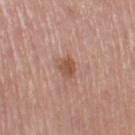Recorded during total-body skin imaging; not selected for excision or biopsy. This is a white-light tile. The lesion's longest dimension is about 2.5 mm. From the left thigh. A female patient, aged 63–67. Cropped from a whole-body photographic skin survey; the tile spans about 15 mm. Automated image analysis of the tile measured an area of roughly 4.5 mm², a shape eccentricity near 0.7, and a symmetry-axis asymmetry near 0.25. The software also gave a lesion–skin lightness drop of about 10 and a lesion-to-skin contrast of about 7 (normalized; higher = more distinct). It also reported border irregularity of about 2 on a 0–10 scale and a peripheral color-asymmetry measure near 1.5. The analysis additionally found a classifier nevus-likeness of about 65/100.